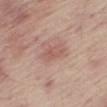Clinical summary: The total-body-photography lesion software estimated an outline eccentricity of about 0.8 (0 = round, 1 = elongated) and a shape-asymmetry score of about 0.25 (0 = symmetric). A 15 mm crop from a total-body photograph taken for skin-cancer surveillance. A male subject approximately 65 years of age. From the right thigh. The lesion's longest dimension is about 3 mm.